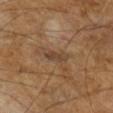biopsy_status: not biopsied; imaged during a skin examination
lighting: cross-polarized
patient:
  sex: male
  age_approx: 65
lesion_size:
  long_diameter_mm_approx: 2.5
image:
  source: total-body photography crop
  field_of_view_mm: 15
automated_metrics:
  area_mm2_approx: 3.0
  eccentricity: 0.85
  shape_asymmetry: 0.25
  lesion_detection_confidence_0_100: 95